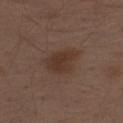This lesion was catalogued during total-body skin photography and was not selected for biopsy. This is a white-light tile. The lesion's longest dimension is about 4.5 mm. Automated image analysis of the tile measured a lesion color around L≈34 a*≈16 b*≈24 in CIELAB. Located on the left thigh. Cropped from a whole-body photographic skin survey; the tile spans about 15 mm. A male patient, about 70 years old.